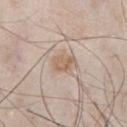Q: Is there a histopathology result?
A: no biopsy performed (imaged during a skin exam)
Q: What did automated image analysis measure?
A: lesion-presence confidence of about 100/100
Q: How was the tile lit?
A: white-light illumination
Q: Where on the body is the lesion?
A: the chest
Q: How large is the lesion?
A: ≈2.5 mm
Q: Who is the patient?
A: male, in their mid-50s
Q: What kind of image is this?
A: ~15 mm tile from a whole-body skin photo